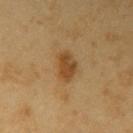Captured during whole-body skin photography for melanoma surveillance; the lesion was not biopsied. The total-body-photography lesion software estimated a shape eccentricity near 0.75 and two-axis asymmetry of about 0.25. The software also gave a within-lesion color-variation index near 3.5/10 and a peripheral color-asymmetry measure near 1. And it measured a classifier nevus-likeness of about 95/100 and a lesion-detection confidence of about 100/100. Cropped from a whole-body photographic skin survey; the tile spans about 15 mm. Located on the right upper arm. A male subject, roughly 60 years of age. Longest diameter approximately 4 mm. The tile uses cross-polarized illumination.